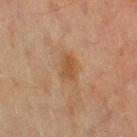Case summary:
– workup · imaged on a skin check; not biopsied
– acquisition · ~15 mm crop, total-body skin-cancer survey
– subject · female, aged approximately 55
– lesion diameter · ~3 mm (longest diameter)
– TBP lesion metrics · a lesion color around L≈45 a*≈18 b*≈31 in CIELAB and a normalized lesion–skin contrast near 6.5
– body site · the leg
– illumination · cross-polarized